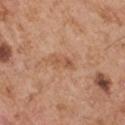Recorded during total-body skin imaging; not selected for excision or biopsy.
About 3 mm across.
This is a white-light tile.
A male subject, roughly 55 years of age.
A lesion tile, about 15 mm wide, cut from a 3D total-body photograph.
The lesion is located on the left upper arm.